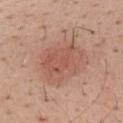Q: Was this lesion biopsied?
A: total-body-photography surveillance lesion; no biopsy
Q: Who is the patient?
A: male, aged 38–42
Q: What is the lesion's diameter?
A: ≈5.5 mm
Q: What is the imaging modality?
A: ~15 mm crop, total-body skin-cancer survey
Q: Where on the body is the lesion?
A: the mid back
Q: What did automated image analysis measure?
A: a lesion area of about 17 mm² and an eccentricity of roughly 0.65; a classifier nevus-likeness of about 60/100 and lesion-presence confidence of about 100/100
Q: What lighting was used for the tile?
A: white-light